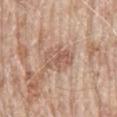No biopsy was performed on this lesion — it was imaged during a full skin examination and was not determined to be concerning. Cropped from a total-body skin-imaging series; the visible field is about 15 mm. From the back. Captured under white-light illumination. The patient is a male roughly 80 years of age.